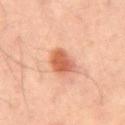The lesion was tiled from a total-body skin photograph and was not biopsied. The lesion's longest dimension is about 3.5 mm. Cropped from a whole-body photographic skin survey; the tile spans about 15 mm. A male patient aged approximately 60. This is a cross-polarized tile. The total-body-photography lesion software estimated border irregularity of about 2 on a 0–10 scale, a color-variation rating of about 3.5/10, and peripheral color asymmetry of about 1.5. Located on the abdomen.